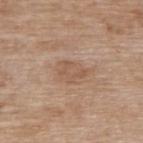Assessment:
No biopsy was performed on this lesion — it was imaged during a full skin examination and was not determined to be concerning.
Image and clinical context:
A female patient aged around 75. The recorded lesion diameter is about 3.5 mm. Automated image analysis of the tile measured a nevus-likeness score of about 0/100 and a lesion-detection confidence of about 100/100. On the upper back. A close-up tile cropped from a whole-body skin photograph, about 15 mm across. Captured under white-light illumination.